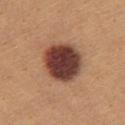Part of a total-body skin-imaging series; this lesion was reviewed on a skin check and was not flagged for biopsy. About 5 mm across. A region of skin cropped from a whole-body photographic capture, roughly 15 mm wide. From the chest. A female patient aged 23 to 27. Imaged with white-light lighting.